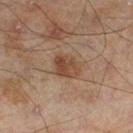Case summary:
• follow-up: catalogued during a skin exam; not biopsied
• diameter: ~3 mm (longest diameter)
• subject: male, aged approximately 45
• body site: the left lower leg
• lighting: cross-polarized illumination
• image-analysis metrics: an area of roughly 7 mm² and an outline eccentricity of about 0.6 (0 = round, 1 = elongated); a border-irregularity index near 2.5/10, internal color variation of about 4 on a 0–10 scale, and radial color variation of about 1.5
• imaging modality: ~15 mm tile from a whole-body skin photo